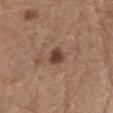– site — the left forearm
– acquisition — total-body-photography crop, ~15 mm field of view
– subject — male, in their 70s